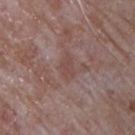Cropped from a whole-body photographic skin survey; the tile spans about 15 mm.
The subject is a male aged around 65.
From the chest.
The lesion-visualizer software estimated an average lesion color of about L≈46 a*≈19 b*≈22 (CIELAB) and a normalized lesion–skin contrast near 5.5. The software also gave a border-irregularity index near 3/10, a within-lesion color-variation index near 1/10, and peripheral color asymmetry of about 0.5. The analysis additionally found a nevus-likeness score of about 0/100 and lesion-presence confidence of about 55/100.
Approximately 3 mm at its widest.